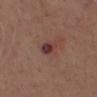Findings:
• workup — imaged on a skin check; not biopsied
• subject — male, roughly 70 years of age
• image source — ~15 mm crop, total-body skin-cancer survey
• location — the chest